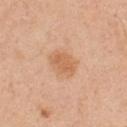<record>
  <site>chest</site>
  <image>
    <source>total-body photography crop</source>
    <field_of_view_mm>15</field_of_view_mm>
  </image>
  <lesion_size>
    <long_diameter_mm_approx>3.5</long_diameter_mm_approx>
  </lesion_size>
  <automated_metrics>
    <area_mm2_approx>8.0</area_mm2_approx>
    <border_irregularity_0_10>1.5</border_irregularity_0_10>
    <color_variation_0_10>2.5</color_variation_0_10>
    <nevus_likeness_0_100>25</nevus_likeness_0_100>
    <lesion_detection_confidence_0_100>100</lesion_detection_confidence_0_100>
  </automated_metrics>
  <lighting>white-light</lighting>
  <patient>
    <sex>male</sex>
    <age_approx>50</age_approx>
  </patient>
</record>